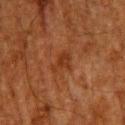Impression:
The lesion was tiled from a total-body skin photograph and was not biopsied.
Image and clinical context:
Located on the upper back. Imaged with cross-polarized lighting. The total-body-photography lesion software estimated an area of roughly 4 mm², a shape eccentricity near 0.7, and a shape-asymmetry score of about 0.55 (0 = symmetric). It also reported a normalized lesion–skin contrast near 6.5. It also reported a lesion-detection confidence of about 100/100. A 15 mm crop from a total-body photograph taken for skin-cancer surveillance. Longest diameter approximately 2.5 mm. A male patient, roughly 60 years of age.